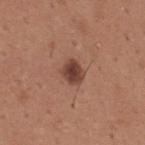The lesion was photographed on a routine skin check and not biopsied; there is no pathology result. A male patient aged 63 to 67. A 15 mm crop from a total-body photograph taken for skin-cancer surveillance. The lesion is on the upper back.